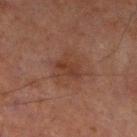This lesion was catalogued during total-body skin photography and was not selected for biopsy. This is a cross-polarized tile. This image is a 15 mm lesion crop taken from a total-body photograph. A male patient aged around 70. The lesion is located on the left lower leg. The lesion's longest dimension is about 3 mm.